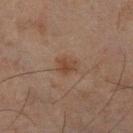Part of a total-body skin-imaging series; this lesion was reviewed on a skin check and was not flagged for biopsy. The subject is a male in their mid-40s. The tile uses cross-polarized illumination. The lesion is on the right lower leg. A lesion tile, about 15 mm wide, cut from a 3D total-body photograph.